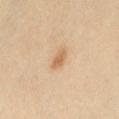{"biopsy_status": "not biopsied; imaged during a skin examination", "lighting": "cross-polarized", "patient": {"sex": "female", "age_approx": 40}, "image": {"source": "total-body photography crop", "field_of_view_mm": 15}, "site": "abdomen", "lesion_size": {"long_diameter_mm_approx": 3.0}, "automated_metrics": {"area_mm2_approx": 3.5, "eccentricity": 0.85, "shape_asymmetry": 0.25, "vs_skin_darker_L": 10.0, "vs_skin_contrast_norm": 6.5, "border_irregularity_0_10": 2.5, "color_variation_0_10": 1.5, "peripheral_color_asymmetry": 0.5}}